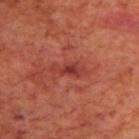Findings:
– notes: catalogued during a skin exam; not biopsied
– subject: male, aged 68–72
– site: the upper back
– tile lighting: cross-polarized illumination
– acquisition: ~15 mm crop, total-body skin-cancer survey